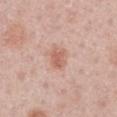The lesion was tiled from a total-body skin photograph and was not biopsied.
A 15 mm crop from a total-body photograph taken for skin-cancer surveillance.
The lesion is on the right upper arm.
The subject is a male about 60 years old.
This is a white-light tile.